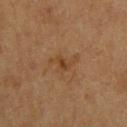biopsy_status: not biopsied; imaged during a skin examination
image:
  source: total-body photography crop
  field_of_view_mm: 15
automated_metrics:
  eccentricity: 0.8
  shape_asymmetry: 0.55
  border_irregularity_0_10: 6.5
  color_variation_0_10: 4.0
  peripheral_color_asymmetry: 1.5
  lesion_detection_confidence_0_100: 100
patient:
  sex: male
  age_approx: 60
lesion_size:
  long_diameter_mm_approx: 3.5